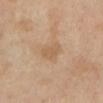The lesion was photographed on a routine skin check and not biopsied; there is no pathology result. On the leg. The patient is a female approximately 70 years of age. This image is a 15 mm lesion crop taken from a total-body photograph.